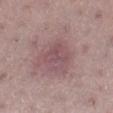Imaged with white-light lighting. A lesion tile, about 15 mm wide, cut from a 3D total-body photograph. On the right lower leg. A female patient, roughly 45 years of age. The recorded lesion diameter is about 3.5 mm.